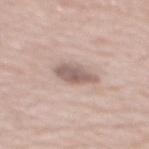A region of skin cropped from a whole-body photographic capture, roughly 15 mm wide.
A female patient aged approximately 65.
The lesion is located on the mid back.